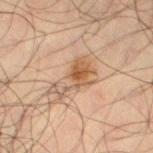Assessment: Captured during whole-body skin photography for melanoma surveillance; the lesion was not biopsied. Image and clinical context: A roughly 15 mm field-of-view crop from a total-body skin photograph. This is a cross-polarized tile. The lesion is on the left thigh. Automated image analysis of the tile measured border irregularity of about 10 on a 0–10 scale and radial color variation of about 1. The analysis additionally found a classifier nevus-likeness of about 85/100 and lesion-presence confidence of about 100/100. A male subject, approximately 35 years of age.